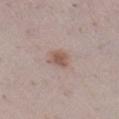The lesion was tiled from a total-body skin photograph and was not biopsied. A female patient in their 30s. Imaged with white-light lighting. The lesion-visualizer software estimated an area of roughly 4.5 mm² and a shape-asymmetry score of about 0.25 (0 = symmetric). The software also gave a lesion color around L≈55 a*≈17 b*≈24 in CIELAB and a normalized lesion–skin contrast near 7.5. It also reported border irregularity of about 2 on a 0–10 scale and internal color variation of about 1.5 on a 0–10 scale. Cropped from a whole-body photographic skin survey; the tile spans about 15 mm. The lesion is on the leg. Approximately 2.5 mm at its widest.